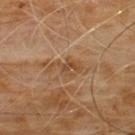The lesion was photographed on a routine skin check and not biopsied; there is no pathology result. The patient is a male approximately 60 years of age. The lesion is on the chest. The lesion's longest dimension is about 4 mm. An algorithmic analysis of the crop reported a lesion area of about 5.5 mm² and a shape-asymmetry score of about 0.45 (0 = symmetric). And it measured a classifier nevus-likeness of about 0/100 and a detector confidence of about 90 out of 100 that the crop contains a lesion. A close-up tile cropped from a whole-body skin photograph, about 15 mm across. Captured under cross-polarized illumination.